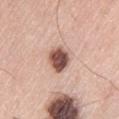This lesion was catalogued during total-body skin photography and was not selected for biopsy. An algorithmic analysis of the crop reported a footprint of about 7.5 mm², a shape eccentricity near 0.65, and a symmetry-axis asymmetry near 0.2. It also reported a mean CIELAB color near L≈53 a*≈22 b*≈25 and a lesion-to-skin contrast of about 12.5 (normalized; higher = more distinct). The analysis additionally found a classifier nevus-likeness of about 90/100. Located on the left thigh. A 15 mm close-up extracted from a 3D total-body photography capture. Longest diameter approximately 3.5 mm. A male subject aged 78–82. The tile uses white-light illumination.